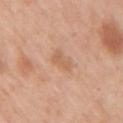The lesion was tiled from a total-body skin photograph and was not biopsied. From the right upper arm. The patient is a female aged around 45. A 15 mm close-up extracted from a 3D total-body photography capture. Measured at roughly 2.5 mm in maximum diameter.